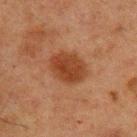biopsy status = catalogued during a skin exam; not biopsied
site = the upper back
patient = male, aged around 60
imaging modality = ~15 mm tile from a whole-body skin photo